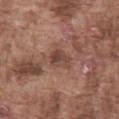workup: imaged on a skin check; not biopsied
anatomic site: the abdomen
lighting: white-light
imaging modality: total-body-photography crop, ~15 mm field of view
patient: male, aged 73 to 77
size: ≈3 mm